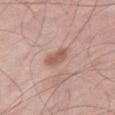Q: Was this lesion biopsied?
A: imaged on a skin check; not biopsied
Q: What is the imaging modality?
A: 15 mm crop, total-body photography
Q: Where on the body is the lesion?
A: the right thigh
Q: How large is the lesion?
A: about 3 mm
Q: What are the patient's age and sex?
A: male, approximately 55 years of age
Q: What lighting was used for the tile?
A: white-light illumination